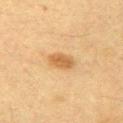Q: Was this lesion biopsied?
A: no biopsy performed (imaged during a skin exam)
Q: What is the imaging modality?
A: ~15 mm crop, total-body skin-cancer survey
Q: What is the anatomic site?
A: the right upper arm
Q: Who is the patient?
A: male, about 55 years old